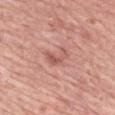<case>
<image>
  <source>total-body photography crop</source>
  <field_of_view_mm>15</field_of_view_mm>
</image>
<automated_metrics>
  <area_mm2_approx>4.0</area_mm2_approx>
  <shape_asymmetry>0.7</shape_asymmetry>
  <cielab_L>56</cielab_L>
  <cielab_a>26</cielab_a>
  <cielab_b>26</cielab_b>
  <vs_skin_darker_L>9.0</vs_skin_darker_L>
  <vs_skin_contrast_norm>6.0</vs_skin_contrast_norm>
</automated_metrics>
<lesion_size>
  <long_diameter_mm_approx>3.0</long_diameter_mm_approx>
</lesion_size>
<lighting>white-light</lighting>
<site>back</site>
<patient>
  <sex>female</sex>
  <age_approx>70</age_approx>
</patient>
</case>